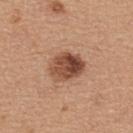This lesion was catalogued during total-body skin photography and was not selected for biopsy.
A close-up tile cropped from a whole-body skin photograph, about 15 mm across.
Measured at roughly 4.5 mm in maximum diameter.
The lesion is located on the upper back.
Captured under white-light illumination.
Automated image analysis of the tile measured an area of roughly 11 mm² and two-axis asymmetry of about 0.15. And it measured a lesion color around L≈48 a*≈23 b*≈31 in CIELAB, roughly 15 lightness units darker than nearby skin, and a normalized border contrast of about 10.5. And it measured a border-irregularity rating of about 1.5/10, a within-lesion color-variation index near 8.5/10, and radial color variation of about 3.5. And it measured an automated nevus-likeness rating near 70 out of 100 and a lesion-detection confidence of about 100/100.
The subject is a male aged 38 to 42.